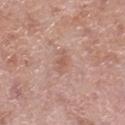Q: Was this lesion biopsied?
A: total-body-photography surveillance lesion; no biopsy
Q: What is the imaging modality?
A: 15 mm crop, total-body photography
Q: What is the anatomic site?
A: the right lower leg
Q: What lighting was used for the tile?
A: white-light illumination
Q: What is the lesion's diameter?
A: about 3 mm
Q: What are the patient's age and sex?
A: male, aged around 70